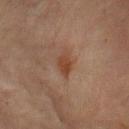notes = catalogued during a skin exam; not biopsied | location = the left upper arm | subject = female, roughly 80 years of age | lesion size = ~3.5 mm (longest diameter) | acquisition = ~15 mm tile from a whole-body skin photo.